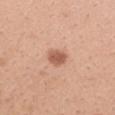Part of a total-body skin-imaging series; this lesion was reviewed on a skin check and was not flagged for biopsy. A female subject approximately 25 years of age. On the right upper arm. Captured under white-light illumination. The lesion's longest dimension is about 2.5 mm. A lesion tile, about 15 mm wide, cut from a 3D total-body photograph.